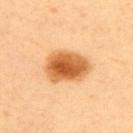Imaged during a routine full-body skin examination; the lesion was not biopsied and no histopathology is available. A roughly 15 mm field-of-view crop from a total-body skin photograph. Located on the back. A female patient, aged approximately 40. Approximately 5.5 mm at its widest.